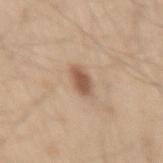Clinical impression: Captured during whole-body skin photography for melanoma surveillance; the lesion was not biopsied. Context: A male patient, approximately 60 years of age. A 15 mm crop from a total-body photograph taken for skin-cancer surveillance. The lesion's longest dimension is about 3 mm. An algorithmic analysis of the crop reported an area of roughly 4 mm², an outline eccentricity of about 0.85 (0 = round, 1 = elongated), and a symmetry-axis asymmetry near 0.25. The software also gave a normalized border contrast of about 8.5. The software also gave a lesion-detection confidence of about 100/100. The lesion is located on the right thigh. Imaged with white-light lighting.